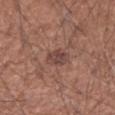Case summary:
– workup — total-body-photography surveillance lesion; no biopsy
– subject — male, aged 58–62
– acquisition — ~15 mm crop, total-body skin-cancer survey
– tile lighting — white-light illumination
– location — the right forearm
– size — about 2.5 mm